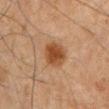workup = total-body-photography surveillance lesion; no biopsy
subject = male, aged 78 to 82
illumination = cross-polarized
site = the abdomen
imaging modality = ~15 mm crop, total-body skin-cancer survey
lesion diameter = ≈3.5 mm